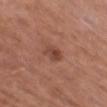<record>
<site>leg</site>
<image>
  <source>total-body photography crop</source>
  <field_of_view_mm>15</field_of_view_mm>
</image>
<lighting>white-light</lighting>
<lesion_size>
  <long_diameter_mm_approx>2.5</long_diameter_mm_approx>
</lesion_size>
<patient>
  <sex>male</sex>
  <age_approx>65</age_approx>
</patient>
</record>